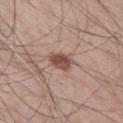Notes:
• workup: imaged on a skin check; not biopsied
• image: ~15 mm tile from a whole-body skin photo
• anatomic site: the left thigh
• diameter: about 3 mm
• patient: male, in their 60s
• automated metrics: a within-lesion color-variation index near 3.5/10 and peripheral color asymmetry of about 1.5; a lesion-detection confidence of about 100/100
• illumination: white-light illumination